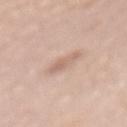notes: no biopsy performed (imaged during a skin exam)
TBP lesion metrics: an eccentricity of roughly 0.9 and a symmetry-axis asymmetry near 0.25; an average lesion color of about L≈64 a*≈18 b*≈27 (CIELAB) and a normalized border contrast of about 5.5; peripheral color asymmetry of about 0.5
lesion diameter: ~3.5 mm (longest diameter)
site: the back
patient: female, approximately 60 years of age
lighting: white-light illumination
acquisition: total-body-photography crop, ~15 mm field of view